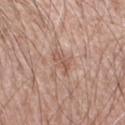Assessment: Captured during whole-body skin photography for melanoma surveillance; the lesion was not biopsied. Acquisition and patient details: About 3 mm across. On the right forearm. A region of skin cropped from a whole-body photographic capture, roughly 15 mm wide. The subject is a male aged 58 to 62. Automated image analysis of the tile measured a shape-asymmetry score of about 0.4 (0 = symmetric). The software also gave border irregularity of about 4.5 on a 0–10 scale, a color-variation rating of about 1.5/10, and a peripheral color-asymmetry measure near 0.5. And it measured a lesion-detection confidence of about 100/100. This is a white-light tile.